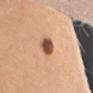<case>
  <biopsy_status>not biopsied; imaged during a skin examination</biopsy_status>
  <image>
    <source>total-body photography crop</source>
    <field_of_view_mm>15</field_of_view_mm>
  </image>
  <site>arm</site>
  <lighting>white-light</lighting>
  <patient>
    <sex>female</sex>
    <age_approx>55</age_approx>
  </patient>
</case>